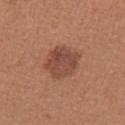Case summary:
* biopsy status: total-body-photography surveillance lesion; no biopsy
* illumination: white-light
* image: ~15 mm tile from a whole-body skin photo
* body site: the arm
* image-analysis metrics: a mean CIELAB color near L≈47 a*≈24 b*≈28 and a lesion–skin lightness drop of about 10; radial color variation of about 1; a nevus-likeness score of about 70/100 and a lesion-detection confidence of about 100/100
* patient: female, approximately 40 years of age